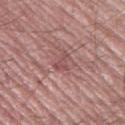Part of a total-body skin-imaging series; this lesion was reviewed on a skin check and was not flagged for biopsy.
The lesion is on the left thigh.
Approximately 2.5 mm at its widest.
A 15 mm crop from a total-body photograph taken for skin-cancer surveillance.
A male subject, aged approximately 60.